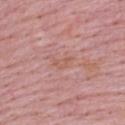The lesion is located on the upper back. A male patient in their mid-60s. A close-up tile cropped from a whole-body skin photograph, about 15 mm across.